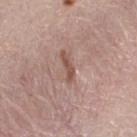- tile lighting · white-light
- patient · male, aged 28–32
- site · the upper back
- diameter · about 2.5 mm
- acquisition · total-body-photography crop, ~15 mm field of view